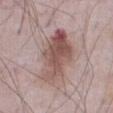lighting: white-light
image:
  source: total-body photography crop
  field_of_view_mm: 15
site: abdomen
patient:
  sex: male
  age_approx: 75